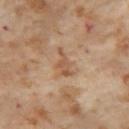Q: Is there a histopathology result?
A: total-body-photography surveillance lesion; no biopsy
Q: What are the patient's age and sex?
A: female, about 55 years old
Q: What lighting was used for the tile?
A: cross-polarized illumination
Q: What kind of image is this?
A: ~15 mm crop, total-body skin-cancer survey
Q: Automated lesion metrics?
A: a lesion color around L≈55 a*≈20 b*≈33 in CIELAB, about 8 CIELAB-L* units darker than the surrounding skin, and a lesion-to-skin contrast of about 6 (normalized; higher = more distinct); an automated nevus-likeness rating near 0 out of 100 and a detector confidence of about 100 out of 100 that the crop contains a lesion
Q: Lesion location?
A: the left thigh
Q: What is the lesion's diameter?
A: ~3.5 mm (longest diameter)